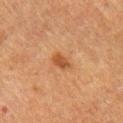follow-up: total-body-photography surveillance lesion; no biopsy.